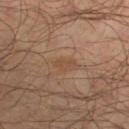The lesion was tiled from a total-body skin photograph and was not biopsied. A 15 mm crop from a total-body photograph taken for skin-cancer surveillance. A male patient, roughly 65 years of age. Automated tile analysis of the lesion measured a footprint of about 3 mm², an outline eccentricity of about 0.85 (0 = round, 1 = elongated), and two-axis asymmetry of about 0.25. And it measured about 5 CIELAB-L* units darker than the surrounding skin and a normalized lesion–skin contrast near 5. And it measured a border-irregularity rating of about 3/10, a within-lesion color-variation index near 1.5/10, and peripheral color asymmetry of about 0.5. The analysis additionally found a classifier nevus-likeness of about 10/100 and a detector confidence of about 100 out of 100 that the crop contains a lesion. On the right lower leg.